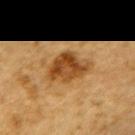Notes:
- follow-up · catalogued during a skin exam; not biopsied
- subject · male, in their mid-80s or older
- anatomic site · the upper back
- image · 15 mm crop, total-body photography
- lighting · cross-polarized
- lesion diameter · ~5.5 mm (longest diameter)